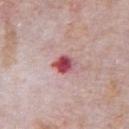Notes:
– biopsy status · catalogued during a skin exam; not biopsied
– anatomic site · the front of the torso
– diameter · ~3 mm (longest diameter)
– image source · ~15 mm tile from a whole-body skin photo
– subject · male, roughly 70 years of age
– tile lighting · white-light